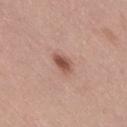Q: Was this lesion biopsied?
A: total-body-photography surveillance lesion; no biopsy
Q: Where on the body is the lesion?
A: the right thigh
Q: What lighting was used for the tile?
A: white-light illumination
Q: What kind of image is this?
A: ~15 mm crop, total-body skin-cancer survey
Q: Patient demographics?
A: female, aged 38–42